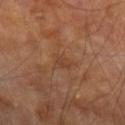This lesion was catalogued during total-body skin photography and was not selected for biopsy. The subject is a male roughly 70 years of age. On the left forearm. Cropped from a total-body skin-imaging series; the visible field is about 15 mm. About 2.5 mm across. This is a cross-polarized tile.